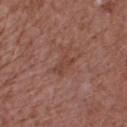{"biopsy_status": "not biopsied; imaged during a skin examination", "image": {"source": "total-body photography crop", "field_of_view_mm": 15}, "site": "chest", "patient": {"sex": "male", "age_approx": 75}, "lesion_size": {"long_diameter_mm_approx": 3.5}, "lighting": "white-light"}